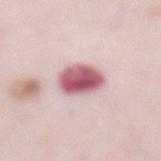Located on the abdomen.
A region of skin cropped from a whole-body photographic capture, roughly 15 mm wide.
A female subject aged approximately 65.